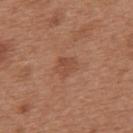Q: Was a biopsy performed?
A: imaged on a skin check; not biopsied
Q: What is the anatomic site?
A: the back
Q: How was the tile lit?
A: white-light
Q: What are the patient's age and sex?
A: female, about 40 years old
Q: What is the lesion's diameter?
A: about 3 mm
Q: How was this image acquired?
A: ~15 mm tile from a whole-body skin photo